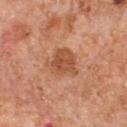Q: Was a biopsy performed?
A: imaged on a skin check; not biopsied
Q: Patient demographics?
A: male, aged approximately 55
Q: Lesion location?
A: the front of the torso
Q: How was this image acquired?
A: ~15 mm tile from a whole-body skin photo